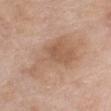| key | value |
|---|---|
| notes | no biopsy performed (imaged during a skin exam) |
| patient | female, aged 73 to 77 |
| site | the front of the torso |
| image source | ~15 mm crop, total-body skin-cancer survey |